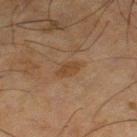Recorded during total-body skin imaging; not selected for excision or biopsy. Automated image analysis of the tile measured an average lesion color of about L≈34 a*≈15 b*≈27 (CIELAB), a lesion–skin lightness drop of about 5, and a normalized border contrast of about 6. A lesion tile, about 15 mm wide, cut from a 3D total-body photograph. The lesion is on the leg. Imaged with cross-polarized lighting. Measured at roughly 3.5 mm in maximum diameter. A male patient, about 65 years old.